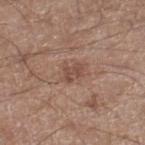Notes:
- notes — total-body-photography surveillance lesion; no biopsy
- tile lighting — white-light
- image-analysis metrics — a footprint of about 4 mm², a shape eccentricity near 0.7, and two-axis asymmetry of about 0.4; an average lesion color of about L≈48 a*≈19 b*≈25 (CIELAB) and a lesion–skin lightness drop of about 8
- lesion size — ~2.5 mm (longest diameter)
- acquisition — ~15 mm crop, total-body skin-cancer survey
- subject — male, aged 58–62
- anatomic site — the right lower leg